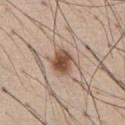Imaged during a routine full-body skin examination; the lesion was not biopsied and no histopathology is available. Cropped from a whole-body photographic skin survey; the tile spans about 15 mm. Automated image analysis of the tile measured a lesion area of about 6.5 mm², an eccentricity of roughly 0.6, and two-axis asymmetry of about 0.2. It also reported an average lesion color of about L≈51 a*≈19 b*≈29 (CIELAB), roughly 15 lightness units darker than nearby skin, and a normalized border contrast of about 10.5. And it measured a classifier nevus-likeness of about 100/100 and a detector confidence of about 100 out of 100 that the crop contains a lesion. Longest diameter approximately 3 mm. A male subject, aged around 55. The lesion is on the chest. The tile uses white-light illumination.